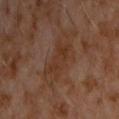Findings:
- tile lighting: cross-polarized
- diameter: about 6 mm
- image-analysis metrics: an outline eccentricity of about 0.85 (0 = round, 1 = elongated) and two-axis asymmetry of about 0.4; a lesion color around L≈32 a*≈17 b*≈26 in CIELAB, about 5 CIELAB-L* units darker than the surrounding skin, and a normalized lesion–skin contrast near 6; a border-irregularity index near 5.5/10, a within-lesion color-variation index near 3/10, and radial color variation of about 1; a classifier nevus-likeness of about 0/100 and a lesion-detection confidence of about 100/100
- location: the front of the torso
- image source: ~15 mm tile from a whole-body skin photo
- patient: male, roughly 60 years of age